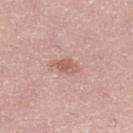workup — no biopsy performed (imaged during a skin exam)
patient — female, approximately 40 years of age
diameter — ≈3 mm
body site — the right lower leg
image source — ~15 mm tile from a whole-body skin photo
illumination — white-light illumination